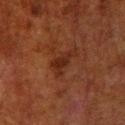Q: Is there a histopathology result?
A: total-body-photography surveillance lesion; no biopsy
Q: What is the imaging modality?
A: ~15 mm tile from a whole-body skin photo
Q: Lesion location?
A: the right lower leg
Q: Lesion size?
A: ≈3.5 mm
Q: What are the patient's age and sex?
A: male, aged 78–82
Q: What did automated image analysis measure?
A: a lesion area of about 5 mm² and an outline eccentricity of about 0.85 (0 = round, 1 = elongated)
Q: What lighting was used for the tile?
A: cross-polarized